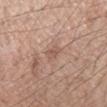Impression: The lesion was tiled from a total-body skin photograph and was not biopsied. Clinical summary: Imaged with white-light lighting. A 15 mm crop from a total-body photograph taken for skin-cancer surveillance. A male patient, approximately 40 years of age. On the left forearm.